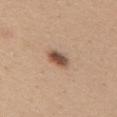The lesion was photographed on a routine skin check and not biopsied; there is no pathology result.
This is a white-light tile.
Automated image analysis of the tile measured an outline eccentricity of about 0.7 (0 = round, 1 = elongated) and a symmetry-axis asymmetry near 0.25. And it measured a mean CIELAB color near L≈51 a*≈19 b*≈28, a lesion–skin lightness drop of about 15, and a normalized border contrast of about 10.5.
On the upper back.
Cropped from a total-body skin-imaging series; the visible field is about 15 mm.
A female patient, about 45 years old.